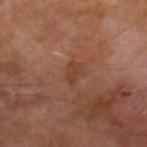Findings:
– biopsy status — no biopsy performed (imaged during a skin exam)
– site — the right lower leg
– patient — male, approximately 70 years of age
– acquisition — ~15 mm crop, total-body skin-cancer survey